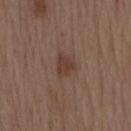Q: Was this lesion biopsied?
A: imaged on a skin check; not biopsied
Q: How was the tile lit?
A: white-light illumination
Q: What kind of image is this?
A: total-body-photography crop, ~15 mm field of view
Q: What are the patient's age and sex?
A: male, aged around 70
Q: What is the anatomic site?
A: the mid back